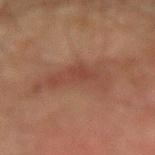Notes:
– workup — no biopsy performed (imaged during a skin exam)
– imaging modality — total-body-photography crop, ~15 mm field of view
– lesion diameter — ≈8 mm
– patient — male, aged approximately 75
– tile lighting — cross-polarized
– location — the right upper arm